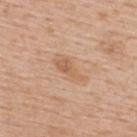This lesion was catalogued during total-body skin photography and was not selected for biopsy.
The tile uses white-light illumination.
The lesion's longest dimension is about 4.5 mm.
The patient is a female roughly 65 years of age.
Cropped from a total-body skin-imaging series; the visible field is about 15 mm.
The lesion is located on the back.